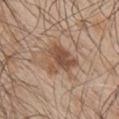<case>
<biopsy_status>not biopsied; imaged during a skin examination</biopsy_status>
<automated_metrics>
  <border_irregularity_0_10>4.0</border_irregularity_0_10>
  <color_variation_0_10>5.5</color_variation_0_10>
  <peripheral_color_asymmetry>2.0</peripheral_color_asymmetry>
</automated_metrics>
<site>arm</site>
<patient>
  <sex>male</sex>
  <age_approx>50</age_approx>
</patient>
<image>
  <source>total-body photography crop</source>
  <field_of_view_mm>15</field_of_view_mm>
</image>
<lighting>white-light</lighting>
</case>